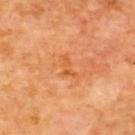follow-up: total-body-photography surveillance lesion; no biopsy | imaging modality: total-body-photography crop, ~15 mm field of view | lesion diameter: ≈3 mm | tile lighting: cross-polarized illumination | subject: male, aged around 60 | site: the back.